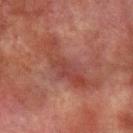workup: imaged on a skin check; not biopsied
site: the arm
subject: male, aged around 75
acquisition: 15 mm crop, total-body photography
automated lesion analysis: border irregularity of about 6.5 on a 0–10 scale and a within-lesion color-variation index near 4.5/10; a classifier nevus-likeness of about 0/100 and a lesion-detection confidence of about 70/100